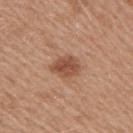Assessment:
This lesion was catalogued during total-body skin photography and was not selected for biopsy.
Image and clinical context:
Cropped from a whole-body photographic skin survey; the tile spans about 15 mm. This is a white-light tile. The lesion is on the right upper arm. The patient is a female about 40 years old. Measured at roughly 3.5 mm in maximum diameter.